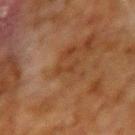Clinical impression: No biopsy was performed on this lesion — it was imaged during a full skin examination and was not determined to be concerning. Context: Approximately 3 mm at its widest. Imaged with cross-polarized lighting. A region of skin cropped from a whole-body photographic capture, roughly 15 mm wide. A male patient approximately 70 years of age. From the arm.